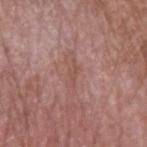Part of a total-body skin-imaging series; this lesion was reviewed on a skin check and was not flagged for biopsy. A 15 mm close-up tile from a total-body photography series done for melanoma screening. The lesion is on the left upper arm. The recorded lesion diameter is about 3 mm. A male subject, in their mid- to late 50s.